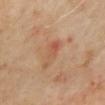Q: Was this lesion biopsied?
A: total-body-photography surveillance lesion; no biopsy